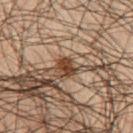| key | value |
|---|---|
| image-analysis metrics | an average lesion color of about L≈29 a*≈16 b*≈24 (CIELAB); a detector confidence of about 100 out of 100 that the crop contains a lesion |
| location | the left upper arm |
| illumination | cross-polarized illumination |
| image | 15 mm crop, total-body photography |
| patient | male, approximately 50 years of age |